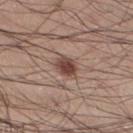Clinical impression:
Part of a total-body skin-imaging series; this lesion was reviewed on a skin check and was not flagged for biopsy.
Image and clinical context:
A 15 mm crop from a total-body photograph taken for skin-cancer surveillance. Captured under white-light illumination. On the left lower leg. A male patient, in their 30s. Measured at roughly 2.5 mm in maximum diameter.